Located on the upper back. Automated image analysis of the tile measured an area of roughly 13 mm² and a shape eccentricity near 0.7. The software also gave a border-irregularity rating of about 3.5/10, internal color variation of about 4.5 on a 0–10 scale, and peripheral color asymmetry of about 1.5. A lesion tile, about 15 mm wide, cut from a 3D total-body photograph. This is a white-light tile. A male subject, aged 28 to 32: 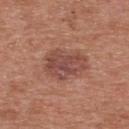| field | value |
|---|---|
| pathology | a dysplastic (Clark) nevus |